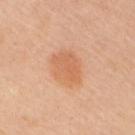| field | value |
|---|---|
| biopsy status | imaged on a skin check; not biopsied |
| automated lesion analysis | an average lesion color of about L≈64 a*≈25 b*≈37 (CIELAB) and about 8 CIELAB-L* units darker than the surrounding skin |
| patient | female, approximately 40 years of age |
| imaging modality | 15 mm crop, total-body photography |
| site | the arm |